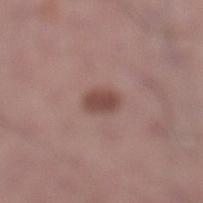No biopsy was performed on this lesion — it was imaged during a full skin examination and was not determined to be concerning. The subject is a male in their mid- to late 30s. The lesion is located on the left lower leg. This is a white-light tile. The lesion-visualizer software estimated an automated nevus-likeness rating near 95 out of 100 and a lesion-detection confidence of about 100/100. Longest diameter approximately 2.5 mm. A roughly 15 mm field-of-view crop from a total-body skin photograph.